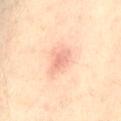The lesion was tiled from a total-body skin photograph and was not biopsied. On the abdomen. Imaged with cross-polarized lighting. A female subject aged approximately 40. A 15 mm close-up tile from a total-body photography series done for melanoma screening.